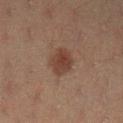No biopsy was performed on this lesion — it was imaged during a full skin examination and was not determined to be concerning.
From the left lower leg.
A close-up tile cropped from a whole-body skin photograph, about 15 mm across.
Automated image analysis of the tile measured a footprint of about 7 mm² and a shape-asymmetry score of about 0.15 (0 = symmetric). The software also gave a border-irregularity rating of about 1.5/10, a color-variation rating of about 2.5/10, and peripheral color asymmetry of about 1. The analysis additionally found a classifier nevus-likeness of about 90/100.
A female patient, aged approximately 20.